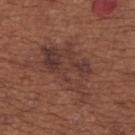  biopsy_status: not biopsied; imaged during a skin examination
  site: upper back
  patient:
    sex: female
    age_approx: 65
  image:
    source: total-body photography crop
    field_of_view_mm: 15